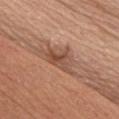Clinical impression:
No biopsy was performed on this lesion — it was imaged during a full skin examination and was not determined to be concerning.
Image and clinical context:
From the front of the torso. About 5 mm across. The tile uses white-light illumination. The patient is a male aged approximately 55. A close-up tile cropped from a whole-body skin photograph, about 15 mm across.